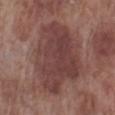Findings:
• notes — catalogued during a skin exam; not biopsied
• lesion size — about 9.5 mm
• image source — total-body-photography crop, ~15 mm field of view
• anatomic site — the leg
• automated metrics — an area of roughly 43 mm², an eccentricity of roughly 0.75, and a shape-asymmetry score of about 0.25 (0 = symmetric); border irregularity of about 3 on a 0–10 scale, a within-lesion color-variation index near 4/10, and a peripheral color-asymmetry measure near 1.5; an automated nevus-likeness rating near 0 out of 100 and a lesion-detection confidence of about 100/100
• subject — male, aged approximately 70